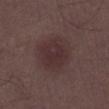Recorded during total-body skin imaging; not selected for excision or biopsy.
A close-up tile cropped from a whole-body skin photograph, about 15 mm across.
Longest diameter approximately 6 mm.
Imaged with white-light lighting.
A male patient, roughly 50 years of age.